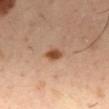This lesion was catalogued during total-body skin photography and was not selected for biopsy. The recorded lesion diameter is about 3 mm. The tile uses cross-polarized illumination. A male subject, aged approximately 40. The lesion-visualizer software estimated a lesion area of about 4 mm², a shape eccentricity near 0.75, and two-axis asymmetry of about 0.2. The software also gave a mean CIELAB color near L≈49 a*≈22 b*≈33, a lesion–skin lightness drop of about 13, and a normalized lesion–skin contrast near 9.5. And it measured border irregularity of about 2 on a 0–10 scale, a within-lesion color-variation index near 3.5/10, and peripheral color asymmetry of about 1. A 15 mm close-up tile from a total-body photography series done for melanoma screening. On the mid back.